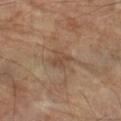The lesion was tiled from a total-body skin photograph and was not biopsied.
The tile uses cross-polarized illumination.
A male subject in their 70s.
Cropped from a total-body skin-imaging series; the visible field is about 15 mm.
The recorded lesion diameter is about 2.5 mm.
Located on the left forearm.